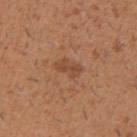Clinical impression: Captured during whole-body skin photography for melanoma surveillance; the lesion was not biopsied. Acquisition and patient details: Cropped from a total-body skin-imaging series; the visible field is about 15 mm. This is a white-light tile. The patient is a male in their 40s. The recorded lesion diameter is about 3 mm. The lesion is located on the right upper arm.